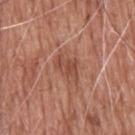The lesion was photographed on a routine skin check and not biopsied; there is no pathology result.
The tile uses white-light illumination.
A male subject aged approximately 60.
The total-body-photography lesion software estimated a footprint of about 5.5 mm², an outline eccentricity of about 0.8 (0 = round, 1 = elongated), and a symmetry-axis asymmetry near 0.35. And it measured a within-lesion color-variation index near 3/10 and radial color variation of about 1. It also reported an automated nevus-likeness rating near 0 out of 100.
Measured at roughly 3.5 mm in maximum diameter.
From the head or neck.
A roughly 15 mm field-of-view crop from a total-body skin photograph.